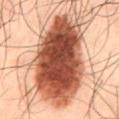workup: catalogued during a skin exam; not biopsied
TBP lesion metrics: a footprint of about 65 mm², a shape eccentricity near 0.9, and two-axis asymmetry of about 0.3; border irregularity of about 6 on a 0–10 scale and a peripheral color-asymmetry measure near 3.5; a lesion-detection confidence of about 100/100
site: the mid back
diameter: ~16.5 mm (longest diameter)
image: ~15 mm tile from a whole-body skin photo
subject: male, aged 48–52